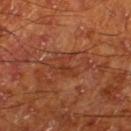<record>
  <patient>
    <sex>male</sex>
    <age_approx>65</age_approx>
  </patient>
  <site>right lower leg</site>
  <image>
    <source>total-body photography crop</source>
    <field_of_view_mm>15</field_of_view_mm>
  </image>
</record>